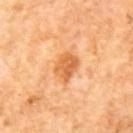body site: the mid back | automated metrics: an outline eccentricity of about 0.75 (0 = round, 1 = elongated) and a symmetry-axis asymmetry near 0.2; an average lesion color of about L≈61 a*≈26 b*≈44 (CIELAB) and roughly 11 lightness units darker than nearby skin | lesion size: ~4 mm (longest diameter) | imaging modality: ~15 mm tile from a whole-body skin photo | patient: male, in their mid- to late 60s.